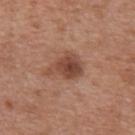  biopsy_status: not biopsied; imaged during a skin examination
  patient:
    sex: female
    age_approx: 35
  lighting: white-light
  automated_metrics:
    cielab_L: 46
    cielab_a: 22
    cielab_b: 28
    vs_skin_darker_L: 11.0
    vs_skin_contrast_norm: 8.5
  image:
    source: total-body photography crop
    field_of_view_mm: 15
  site: back